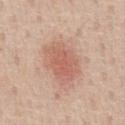The lesion was tiled from a total-body skin photograph and was not biopsied. A male patient, aged 58–62. The lesion is located on the chest. This image is a 15 mm lesion crop taken from a total-body photograph. Captured under white-light illumination.